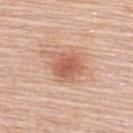follow-up — total-body-photography surveillance lesion; no biopsy | lighting — white-light illumination | anatomic site — the upper back | imaging modality — 15 mm crop, total-body photography | patient — female, aged around 65.